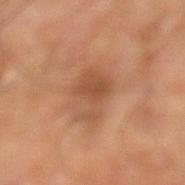The lesion was photographed on a routine skin check and not biopsied; there is no pathology result. A male patient, aged 68–72. Measured at roughly 4.5 mm in maximum diameter. From the left lower leg. This image is a 15 mm lesion crop taken from a total-body photograph.